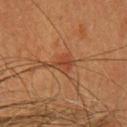workup=catalogued during a skin exam; not biopsied | image source=total-body-photography crop, ~15 mm field of view | image-analysis metrics=a lesion color around L≈37 a*≈22 b*≈30 in CIELAB and a normalized border contrast of about 6; a border-irregularity index near 2.5/10, internal color variation of about 3.5 on a 0–10 scale, and peripheral color asymmetry of about 1.5; an automated nevus-likeness rating near 0 out of 100 and lesion-presence confidence of about 90/100 | location=the head or neck | subject=female, in their 40s | lesion diameter=about 2.5 mm | tile lighting=cross-polarized.